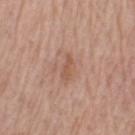Part of a total-body skin-imaging series; this lesion was reviewed on a skin check and was not flagged for biopsy.
On the arm.
The tile uses white-light illumination.
Cropped from a whole-body photographic skin survey; the tile spans about 15 mm.
A male patient, about 65 years old.
Approximately 3 mm at its widest.
The total-body-photography lesion software estimated a footprint of about 2.5 mm², a shape eccentricity near 0.95, and two-axis asymmetry of about 0.4. The software also gave an average lesion color of about L≈54 a*≈21 b*≈30 (CIELAB) and a normalized border contrast of about 6.